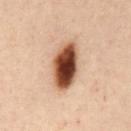No biopsy was performed on this lesion — it was imaged during a full skin examination and was not determined to be concerning. On the abdomen. A 15 mm crop from a total-body photograph taken for skin-cancer surveillance. About 5 mm across. The subject is a female about 55 years old.